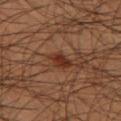Q: Is there a histopathology result?
A: total-body-photography surveillance lesion; no biopsy
Q: Lesion location?
A: the left thigh
Q: How was the tile lit?
A: cross-polarized
Q: What kind of image is this?
A: ~15 mm tile from a whole-body skin photo
Q: What is the lesion's diameter?
A: ≈2.5 mm
Q: What are the patient's age and sex?
A: male, in their mid-40s